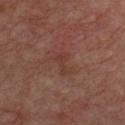{
  "biopsy_status": "not biopsied; imaged during a skin examination",
  "site": "front of the torso",
  "automated_metrics": {
    "area_mm2_approx": 4.5,
    "eccentricity": 0.85,
    "cielab_L": 36,
    "cielab_a": 21,
    "cielab_b": 24,
    "vs_skin_darker_L": 5.0,
    "vs_skin_contrast_norm": 5.0,
    "nevus_likeness_0_100": 0,
    "lesion_detection_confidence_0_100": 100
  },
  "patient": {
    "sex": "male",
    "age_approx": 65
  },
  "lighting": "cross-polarized",
  "image": {
    "source": "total-body photography crop",
    "field_of_view_mm": 15
  },
  "lesion_size": {
    "long_diameter_mm_approx": 3.0
  }
}